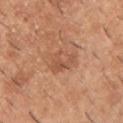Impression: This lesion was catalogued during total-body skin photography and was not selected for biopsy. Clinical summary: A 15 mm crop from a total-body photograph taken for skin-cancer surveillance. Captured under white-light illumination. The total-body-photography lesion software estimated a border-irregularity index near 3.5/10, a color-variation rating of about 4.5/10, and radial color variation of about 1.5. It also reported a lesion-detection confidence of about 100/100. Located on the chest. A male subject aged 38 to 42.